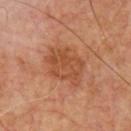Q: Was this lesion biopsied?
A: total-body-photography surveillance lesion; no biopsy
Q: What is the imaging modality?
A: 15 mm crop, total-body photography
Q: Automated lesion metrics?
A: a mean CIELAB color near L≈47 a*≈24 b*≈34, roughly 8 lightness units darker than nearby skin, and a normalized lesion–skin contrast near 6.5; a nevus-likeness score of about 5/100
Q: Lesion size?
A: ~5 mm (longest diameter)
Q: Patient demographics?
A: male, approximately 65 years of age
Q: How was the tile lit?
A: cross-polarized illumination
Q: Where on the body is the lesion?
A: the chest